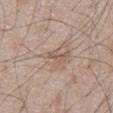Impression: Imaged during a routine full-body skin examination; the lesion was not biopsied and no histopathology is available. Context: The patient is a male about 50 years old. An algorithmic analysis of the crop reported a lesion area of about 2.5 mm² and an eccentricity of roughly 0.9. The software also gave a within-lesion color-variation index near 0/10 and radial color variation of about 0. The software also gave a classifier nevus-likeness of about 0/100 and a lesion-detection confidence of about 55/100. A close-up tile cropped from a whole-body skin photograph, about 15 mm across. On the abdomen.